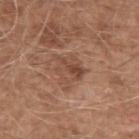| key | value |
|---|---|
| follow-up | total-body-photography surveillance lesion; no biopsy |
| anatomic site | the right upper arm |
| tile lighting | white-light illumination |
| diameter | ~4 mm (longest diameter) |
| imaging modality | 15 mm crop, total-body photography |
| subject | male, about 60 years old |